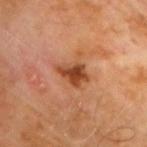follow-up: imaged on a skin check; not biopsied | location: the arm | acquisition: 15 mm crop, total-body photography | TBP lesion metrics: an average lesion color of about L≈44 a*≈27 b*≈36 (CIELAB), about 13 CIELAB-L* units darker than the surrounding skin, and a normalized border contrast of about 10; border irregularity of about 3.5 on a 0–10 scale and a within-lesion color-variation index near 3.5/10; a lesion-detection confidence of about 100/100 | diameter: ~3.5 mm (longest diameter) | lighting: cross-polarized illumination | subject: male, aged 58 to 62.